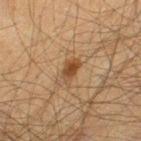Captured during whole-body skin photography for melanoma surveillance; the lesion was not biopsied.
A roughly 15 mm field-of-view crop from a total-body skin photograph.
Located on the left thigh.
A male patient aged around 50.